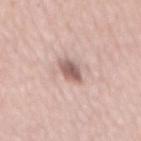Q: How was the tile lit?
A: white-light
Q: Where on the body is the lesion?
A: the mid back
Q: Lesion size?
A: about 3 mm
Q: What is the imaging modality?
A: ~15 mm crop, total-body skin-cancer survey
Q: What are the patient's age and sex?
A: female, aged 63–67